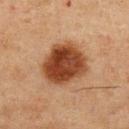Imaged during a routine full-body skin examination; the lesion was not biopsied and no histopathology is available. Automated tile analysis of the lesion measured a border-irregularity index near 1.5/10, a within-lesion color-variation index near 5/10, and peripheral color asymmetry of about 1.5. And it measured a classifier nevus-likeness of about 100/100. Approximately 5.5 mm at its widest. On the abdomen. A region of skin cropped from a whole-body photographic capture, roughly 15 mm wide. Captured under cross-polarized illumination. A male subject, aged around 75.